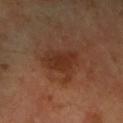Case summary:
- illumination: cross-polarized
- imaging modality: total-body-photography crop, ~15 mm field of view
- body site: the right forearm
- size: ≈4.5 mm
- subject: female, in their mid- to late 60s